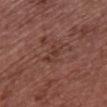<lesion>
<biopsy_status>not biopsied; imaged during a skin examination</biopsy_status>
<lighting>white-light</lighting>
<lesion_size>
  <long_diameter_mm_approx>3.0</long_diameter_mm_approx>
</lesion_size>
<site>chest</site>
<image>
  <source>total-body photography crop</source>
  <field_of_view_mm>15</field_of_view_mm>
</image>
<patient>
  <sex>female</sex>
  <age_approx>65</age_approx>
</patient>
<automated_metrics>
  <area_mm2_approx>5.0</area_mm2_approx>
  <eccentricity>0.85</eccentricity>
  <shape_asymmetry>0.25</shape_asymmetry>
  <cielab_L>37</cielab_L>
  <cielab_a>21</cielab_a>
  <cielab_b>24</cielab_b>
  <nevus_likeness_0_100>0</nevus_likeness_0_100>
</automated_metrics>
</lesion>